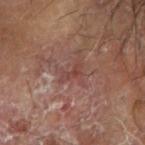Findings:
– workup: catalogued during a skin exam; not biopsied
– subject: male, aged 63–67
– automated metrics: border irregularity of about 6.5 on a 0–10 scale, internal color variation of about 0 on a 0–10 scale, and radial color variation of about 0; a classifier nevus-likeness of about 0/100
– image source: ~15 mm crop, total-body skin-cancer survey
– site: the right forearm